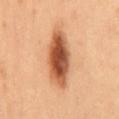Impression:
Imaged during a routine full-body skin examination; the lesion was not biopsied and no histopathology is available.
Clinical summary:
A female subject, about 50 years old. The lesion-visualizer software estimated border irregularity of about 2 on a 0–10 scale and a peripheral color-asymmetry measure near 2.5. A roughly 15 mm field-of-view crop from a total-body skin photograph. Captured under cross-polarized illumination. Located on the back. About 7.5 mm across.